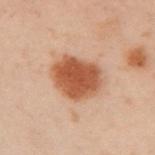{"biopsy_status": "not biopsied; imaged during a skin examination", "site": "left arm", "patient": {"sex": "male", "age_approx": 50}, "lighting": "cross-polarized", "image": {"source": "total-body photography crop", "field_of_view_mm": 15}}